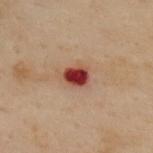workup = catalogued during a skin exam; not biopsied | location = the upper back | patient = female, in their 60s | image = ~15 mm crop, total-body skin-cancer survey | lighting = cross-polarized.